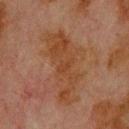Case summary:
– biopsy status · no biopsy performed (imaged during a skin exam)
– tile lighting · cross-polarized
– automated metrics · a lesion color around L≈34 a*≈18 b*≈27 in CIELAB, roughly 5 lightness units darker than nearby skin, and a lesion-to-skin contrast of about 7 (normalized; higher = more distinct); a border-irregularity rating of about 6/10 and a within-lesion color-variation index near 3/10
– image source · ~15 mm tile from a whole-body skin photo
– lesion size · ~8.5 mm (longest diameter)
– location · the upper back
– patient · male, roughly 80 years of age